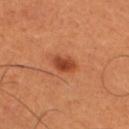{
  "biopsy_status": "not biopsied; imaged during a skin examination",
  "patient": {
    "sex": "male",
    "age_approx": 50
  },
  "image": {
    "source": "total-body photography crop",
    "field_of_view_mm": 15
  },
  "site": "leg"
}